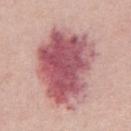Assessment: Imaged during a routine full-body skin examination; the lesion was not biopsied and no histopathology is available. Image and clinical context: A region of skin cropped from a whole-body photographic capture, roughly 15 mm wide. On the chest. Approximately 9.5 mm at its widest. The subject is a male in their mid- to late 50s. Automated image analysis of the tile measured about 16 CIELAB-L* units darker than the surrounding skin and a normalized border contrast of about 10.5. The software also gave an automated nevus-likeness rating near 35 out of 100 and a lesion-detection confidence of about 100/100. Captured under white-light illumination.